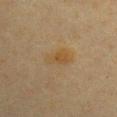No biopsy was performed on this lesion — it was imaged during a full skin examination and was not determined to be concerning.
Captured under cross-polarized illumination.
The subject is a female in their 40s.
Automated image analysis of the tile measured a footprint of about 6 mm², an outline eccentricity of about 0.85 (0 = round, 1 = elongated), and two-axis asymmetry of about 0.25.
On the chest.
Approximately 3.5 mm at its widest.
A close-up tile cropped from a whole-body skin photograph, about 15 mm across.